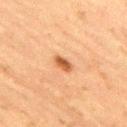Captured during whole-body skin photography for melanoma surveillance; the lesion was not biopsied. The tile uses cross-polarized illumination. An algorithmic analysis of the crop reported an outline eccentricity of about 0.8 (0 = round, 1 = elongated) and a symmetry-axis asymmetry near 0.35. The analysis additionally found a classifier nevus-likeness of about 100/100 and lesion-presence confidence of about 100/100. Cropped from a total-body skin-imaging series; the visible field is about 15 mm. Approximately 2.5 mm at its widest. A male patient, approximately 75 years of age. From the right thigh.